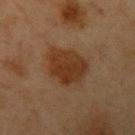<case>
  <biopsy_status>not biopsied; imaged during a skin examination</biopsy_status>
  <site>left upper arm</site>
  <image>
    <source>total-body photography crop</source>
    <field_of_view_mm>15</field_of_view_mm>
  </image>
  <lesion_size>
    <long_diameter_mm_approx>5.5</long_diameter_mm_approx>
  </lesion_size>
  <automated_metrics>
    <border_irregularity_0_10>2.5</border_irregularity_0_10>
    <peripheral_color_asymmetry>1.0</peripheral_color_asymmetry>
    <lesion_detection_confidence_0_100>100</lesion_detection_confidence_0_100>
  </automated_metrics>
  <lighting>cross-polarized</lighting>
  <patient>
    <sex>female</sex>
    <age_approx>40</age_approx>
  </patient>
</case>